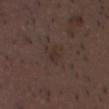Clinical impression:
The lesion was photographed on a routine skin check and not biopsied; there is no pathology result.
Image and clinical context:
An algorithmic analysis of the crop reported an area of roughly 4 mm², an eccentricity of roughly 0.45, and a symmetry-axis asymmetry near 0.4. The analysis additionally found a within-lesion color-variation index near 1/10 and peripheral color asymmetry of about 0.5. The analysis additionally found an automated nevus-likeness rating near 0 out of 100 and a lesion-detection confidence of about 100/100. The patient is a male aged 48–52. A region of skin cropped from a whole-body photographic capture, roughly 15 mm wide. This is a white-light tile. Longest diameter approximately 2.5 mm. The lesion is located on the head or neck.